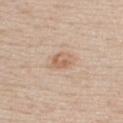  biopsy_status: not biopsied; imaged during a skin examination
  patient:
    sex: male
    age_approx: 55
  site: chest
  image:
    source: total-body photography crop
    field_of_view_mm: 15